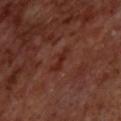{
  "biopsy_status": "not biopsied; imaged during a skin examination",
  "image": {
    "source": "total-body photography crop",
    "field_of_view_mm": 15
  },
  "lighting": "cross-polarized",
  "automated_metrics": {
    "area_mm2_approx": 3.0,
    "eccentricity": 0.95,
    "shape_asymmetry": 0.35,
    "cielab_L": 25,
    "cielab_a": 24,
    "cielab_b": 25,
    "vs_skin_darker_L": 6.0,
    "vs_skin_contrast_norm": 7.0
  },
  "site": "upper back",
  "patient": {
    "sex": "male",
    "age_approx": 70
  }
}